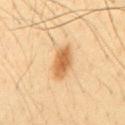workup = imaged on a skin check; not biopsied
image = total-body-photography crop, ~15 mm field of view
diameter = ~4.5 mm (longest diameter)
patient = male, approximately 45 years of age
tile lighting = cross-polarized
body site = the mid back